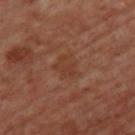follow-up: total-body-photography surveillance lesion; no biopsy
lesion diameter: about 3 mm
subject: female, aged around 45
lighting: cross-polarized
image-analysis metrics: an area of roughly 7 mm² and a shape-asymmetry score of about 0.3 (0 = symmetric); roughly 5 lightness units darker than nearby skin and a normalized border contrast of about 5; a within-lesion color-variation index near 2/10 and peripheral color asymmetry of about 0.5
anatomic site: the upper back
acquisition: ~15 mm tile from a whole-body skin photo